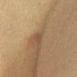notes = imaged on a skin check; not biopsied
imaging modality = 15 mm crop, total-body photography
tile lighting = cross-polarized illumination
patient = female, about 55 years old
lesion diameter = about 2.5 mm
site = the front of the torso
image-analysis metrics = a lesion–skin lightness drop of about 6 and a normalized border contrast of about 5.5